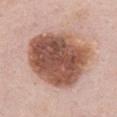Recorded during total-body skin imaging; not selected for excision or biopsy.
A female patient, aged around 60.
An algorithmic analysis of the crop reported peripheral color asymmetry of about 2.5.
Located on the front of the torso.
A 15 mm close-up tile from a total-body photography series done for melanoma screening.
Approximately 8.5 mm at its widest.